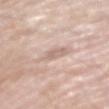Case summary:
• follow-up — catalogued during a skin exam; not biopsied
• patient — male, about 65 years old
• body site — the left upper arm
• image source — ~15 mm crop, total-body skin-cancer survey
• automated metrics — two-axis asymmetry of about 0.25; a border-irregularity rating of about 3/10 and radial color variation of about 1; an automated nevus-likeness rating near 0 out of 100 and a lesion-detection confidence of about 95/100
• lesion diameter — ~4 mm (longest diameter)
• lighting — white-light illumination